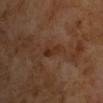Q: Was this lesion biopsied?
A: no biopsy performed (imaged during a skin exam)
Q: Patient demographics?
A: male, about 65 years old
Q: What lighting was used for the tile?
A: cross-polarized illumination
Q: What is the imaging modality?
A: total-body-photography crop, ~15 mm field of view
Q: What is the lesion's diameter?
A: about 3.5 mm